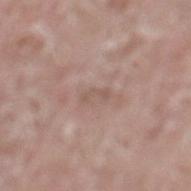Part of a total-body skin-imaging series; this lesion was reviewed on a skin check and was not flagged for biopsy. The lesion is located on the left lower leg. A roughly 15 mm field-of-view crop from a total-body skin photograph. This is a white-light tile. The lesion's longest dimension is about 2.5 mm. The subject is a male about 75 years old. The total-body-photography lesion software estimated an area of roughly 2.5 mm², an outline eccentricity of about 0.9 (0 = round, 1 = elongated), and a shape-asymmetry score of about 0.55 (0 = symmetric). The software also gave a lesion color around L≈55 a*≈17 b*≈24 in CIELAB, roughly 6 lightness units darker than nearby skin, and a lesion-to-skin contrast of about 4.5 (normalized; higher = more distinct). The software also gave a nevus-likeness score of about 0/100 and a detector confidence of about 100 out of 100 that the crop contains a lesion.